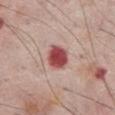notes = catalogued during a skin exam; not biopsied
anatomic site = the front of the torso
automated lesion analysis = a lesion color around L≈49 a*≈32 b*≈23 in CIELAB and a normalized border contrast of about 12.5; a border-irregularity index near 1/10, a color-variation rating of about 3.5/10, and a peripheral color-asymmetry measure near 1; an automated nevus-likeness rating near 5 out of 100 and lesion-presence confidence of about 100/100
illumination = white-light illumination
image source = ~15 mm tile from a whole-body skin photo
subject = male, in their mid-70s
lesion size = ≈3 mm